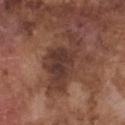Acquisition and patient details:
The recorded lesion diameter is about 7.5 mm. Located on the front of the torso. This image is a 15 mm lesion crop taken from a total-body photograph. This is a white-light tile. Automated image analysis of the tile measured a normalized lesion–skin contrast near 9. It also reported an automated nevus-likeness rating near 0 out of 100. A male patient, approximately 75 years of age.